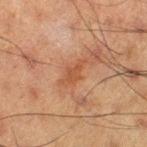Q: Was this lesion biopsied?
A: total-body-photography surveillance lesion; no biopsy
Q: Who is the patient?
A: male, approximately 60 years of age
Q: What is the imaging modality?
A: ~15 mm tile from a whole-body skin photo
Q: Automated lesion metrics?
A: a footprint of about 4 mm² and an eccentricity of roughly 0.9; a nevus-likeness score of about 0/100 and a lesion-detection confidence of about 100/100
Q: Where on the body is the lesion?
A: the left thigh